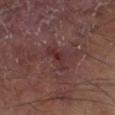biopsy status — total-body-photography surveillance lesion; no biopsy
site — the right lower leg
image source — total-body-photography crop, ~15 mm field of view
subject — male, aged approximately 55